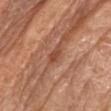follow-up: total-body-photography surveillance lesion; no biopsy
tile lighting: white-light illumination
image: total-body-photography crop, ~15 mm field of view
subject: male, aged 68 to 72
size: about 2.5 mm
location: the left upper arm